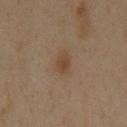Findings:
- patient: male, approximately 60 years of age
- acquisition: 15 mm crop, total-body photography
- site: the front of the torso
- lesion size: about 2.5 mm
- lighting: cross-polarized illumination